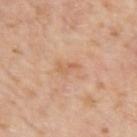Notes:
– biopsy status · total-body-photography surveillance lesion; no biopsy
– automated metrics · a footprint of about 2 mm² and two-axis asymmetry of about 0.5; an average lesion color of about L≈62 a*≈23 b*≈35 (CIELAB)
– patient · male, aged 73–77
– anatomic site · the left upper arm
– image · ~15 mm crop, total-body skin-cancer survey
– lighting · white-light illumination
– lesion diameter · ~3 mm (longest diameter)